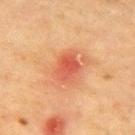Part of a total-body skin-imaging series; this lesion was reviewed on a skin check and was not flagged for biopsy.
The lesion is on the front of the torso.
Cropped from a total-body skin-imaging series; the visible field is about 15 mm.
The recorded lesion diameter is about 3.5 mm.
A male subject, about 85 years old or older.
This is a cross-polarized tile.